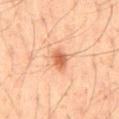Assessment: Part of a total-body skin-imaging series; this lesion was reviewed on a skin check and was not flagged for biopsy. Clinical summary: From the abdomen. A male patient roughly 70 years of age. A 15 mm crop from a total-body photograph taken for skin-cancer surveillance. An algorithmic analysis of the crop reported a classifier nevus-likeness of about 95/100 and a detector confidence of about 100 out of 100 that the crop contains a lesion. The tile uses cross-polarized illumination.